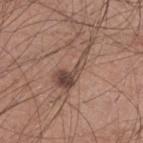Clinical impression: Recorded during total-body skin imaging; not selected for excision or biopsy. Image and clinical context: The tile uses white-light illumination. About 6.5 mm across. A 15 mm close-up extracted from a 3D total-body photography capture. A male subject, aged around 30. The lesion-visualizer software estimated a border-irregularity rating of about 9.5/10, a color-variation rating of about 4.5/10, and radial color variation of about 1.5. The lesion is located on the left lower leg.